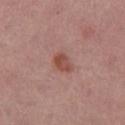Captured during whole-body skin photography for melanoma surveillance; the lesion was not biopsied.
Located on the right thigh.
A close-up tile cropped from a whole-body skin photograph, about 15 mm across.
A female patient, about 55 years old.
Imaged with white-light lighting.
About 2.5 mm across.